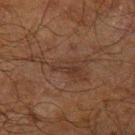No biopsy was performed on this lesion — it was imaged during a full skin examination and was not determined to be concerning. Measured at roughly 7.5 mm in maximum diameter. Cropped from a total-body skin-imaging series; the visible field is about 15 mm. The subject is a male about 70 years old. From the arm. The tile uses cross-polarized illumination. An algorithmic analysis of the crop reported a mean CIELAB color near L≈29 a*≈15 b*≈22 and a normalized border contrast of about 5. It also reported an automated nevus-likeness rating near 0 out of 100 and a lesion-detection confidence of about 60/100.